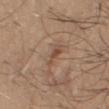| feature | finding |
|---|---|
| follow-up | imaged on a skin check; not biopsied |
| diameter | ~3 mm (longest diameter) |
| tile lighting | white-light illumination |
| patient | male, aged 43 to 47 |
| image source | 15 mm crop, total-body photography |
| image-analysis metrics | roughly 8 lightness units darker than nearby skin and a lesion-to-skin contrast of about 6 (normalized; higher = more distinct) |
| site | the arm |